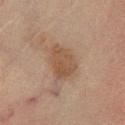Context:
A male subject aged around 65. Imaged with cross-polarized lighting. Automated image analysis of the tile measured a mean CIELAB color near L≈41 a*≈15 b*≈25 and a lesion–skin lightness drop of about 7. It also reported a border-irregularity index near 2.5/10. It also reported a classifier nevus-likeness of about 30/100 and a detector confidence of about 100 out of 100 that the crop contains a lesion. On the left lower leg. Cropped from a whole-body photographic skin survey; the tile spans about 15 mm.